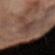{"biopsy_status": "not biopsied; imaged during a skin examination", "patient": {"sex": "female", "age_approx": 50}, "image": {"source": "total-body photography crop", "field_of_view_mm": 15}, "site": "right forearm"}